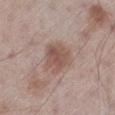The lesion was tiled from a total-body skin photograph and was not biopsied.
From the left lower leg.
The patient is a male aged 58 to 62.
A 15 mm close-up extracted from a 3D total-body photography capture.